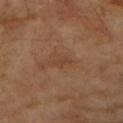  biopsy_status: not biopsied; imaged during a skin examination
  automated_metrics:
    nevus_likeness_0_100: 0
    lesion_detection_confidence_0_100: 100
  site: left forearm
  lighting: cross-polarized
  patient:
    sex: female
    age_approx: 70
  image:
    source: total-body photography crop
    field_of_view_mm: 15